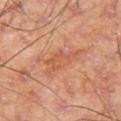Recorded during total-body skin imaging; not selected for excision or biopsy. Measured at roughly 5 mm in maximum diameter. An algorithmic analysis of the crop reported a classifier nevus-likeness of about 0/100 and a detector confidence of about 100 out of 100 that the crop contains a lesion. Imaged with cross-polarized lighting. This image is a 15 mm lesion crop taken from a total-body photograph. From the left thigh. The subject is a male aged approximately 60.